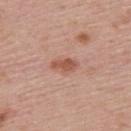Recorded during total-body skin imaging; not selected for excision or biopsy. The subject is a male aged 53–57. Longest diameter approximately 3 mm. Cropped from a whole-body photographic skin survey; the tile spans about 15 mm. This is a white-light tile. The lesion is located on the upper back. An algorithmic analysis of the crop reported a lesion color around L≈54 a*≈24 b*≈30 in CIELAB, a lesion–skin lightness drop of about 11, and a lesion-to-skin contrast of about 7.5 (normalized; higher = more distinct). The analysis additionally found a border-irregularity rating of about 3/10, internal color variation of about 1.5 on a 0–10 scale, and a peripheral color-asymmetry measure near 0.5.